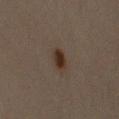Notes:
- workup — catalogued during a skin exam; not biopsied
- image source — ~15 mm crop, total-body skin-cancer survey
- site — the right upper arm
- TBP lesion metrics — an eccentricity of roughly 0.7 and two-axis asymmetry of about 0.15; an automated nevus-likeness rating near 100 out of 100
- subject — female, aged around 45
- lesion diameter — ≈2.5 mm